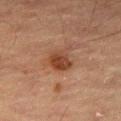Q: Was this lesion biopsied?
A: imaged on a skin check; not biopsied
Q: What did automated image analysis measure?
A: a mean CIELAB color near L≈34 a*≈20 b*≈27 and about 9 CIELAB-L* units darker than the surrounding skin; a border-irregularity index near 2/10, a color-variation rating of about 3/10, and radial color variation of about 1
Q: What lighting was used for the tile?
A: cross-polarized
Q: Lesion location?
A: the left thigh
Q: How large is the lesion?
A: about 3 mm
Q: What is the imaging modality?
A: total-body-photography crop, ~15 mm field of view
Q: Patient demographics?
A: male, about 65 years old